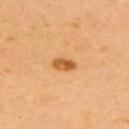  biopsy_status: not biopsied; imaged during a skin examination
  image:
    source: total-body photography crop
    field_of_view_mm: 15
  patient:
    sex: female
    age_approx: 60
  site: upper back